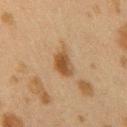{"image": {"source": "total-body photography crop", "field_of_view_mm": 15}, "lighting": "cross-polarized", "site": "right upper arm", "patient": {"sex": "female", "age_approx": 40}}